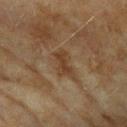This lesion was catalogued during total-body skin photography and was not selected for biopsy. Measured at roughly 4 mm in maximum diameter. From the left upper arm. The subject is a female aged approximately 60. Imaged with cross-polarized lighting. Cropped from a total-body skin-imaging series; the visible field is about 15 mm. The lesion-visualizer software estimated an area of roughly 6.5 mm², a shape eccentricity near 0.85, and a shape-asymmetry score of about 0.4 (0 = symmetric).